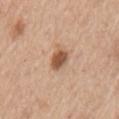Clinical impression:
No biopsy was performed on this lesion — it was imaged during a full skin examination and was not determined to be concerning.
Acquisition and patient details:
Measured at roughly 2.5 mm in maximum diameter. The subject is a male aged approximately 75. A lesion tile, about 15 mm wide, cut from a 3D total-body photograph. Captured under white-light illumination. The lesion is located on the left upper arm.